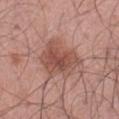Assessment: No biopsy was performed on this lesion — it was imaged during a full skin examination and was not determined to be concerning. Background: The total-body-photography lesion software estimated a lesion color around L≈51 a*≈23 b*≈26 in CIELAB and a lesion–skin lightness drop of about 10. The analysis additionally found a border-irregularity index near 3.5/10 and a within-lesion color-variation index near 5/10. It also reported a nevus-likeness score of about 60/100 and a detector confidence of about 100 out of 100 that the crop contains a lesion. From the front of the torso. A 15 mm close-up tile from a total-body photography series done for melanoma screening. A male subject, aged 68 to 72. Longest diameter approximately 5 mm.